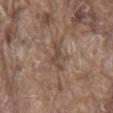{
  "biopsy_status": "not biopsied; imaged during a skin examination",
  "automated_metrics": {
    "shape_asymmetry": 0.45,
    "cielab_L": 46,
    "cielab_a": 16,
    "cielab_b": 25,
    "vs_skin_darker_L": 8.0,
    "vs_skin_contrast_norm": 6.5,
    "border_irregularity_0_10": 6.0,
    "color_variation_0_10": 0.5,
    "nevus_likeness_0_100": 0,
    "lesion_detection_confidence_0_100": 75
  },
  "patient": {
    "sex": "male",
    "age_approx": 80
  },
  "image": {
    "source": "total-body photography crop",
    "field_of_view_mm": 15
  },
  "lesion_size": {
    "long_diameter_mm_approx": 4.0
  },
  "site": "mid back",
  "lighting": "white-light"
}